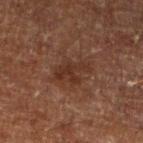Q: Was a biopsy performed?
A: no biopsy performed (imaged during a skin exam)
Q: What is the lesion's diameter?
A: ≈4.5 mm
Q: Who is the patient?
A: male, aged around 60
Q: Where on the body is the lesion?
A: the left lower leg
Q: How was the tile lit?
A: cross-polarized
Q: What kind of image is this?
A: 15 mm crop, total-body photography